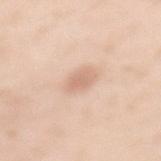Clinical impression: This lesion was catalogued during total-body skin photography and was not selected for biopsy. Context: Measured at roughly 3 mm in maximum diameter. The total-body-photography lesion software estimated a lesion color around L≈69 a*≈20 b*≈30 in CIELAB, roughly 9 lightness units darker than nearby skin, and a normalized lesion–skin contrast near 5.5. The analysis additionally found a border-irregularity index near 1.5/10, a color-variation rating of about 2/10, and radial color variation of about 0.5. It also reported an automated nevus-likeness rating near 75 out of 100 and lesion-presence confidence of about 100/100. The patient is a female approximately 40 years of age. On the mid back. A region of skin cropped from a whole-body photographic capture, roughly 15 mm wide.